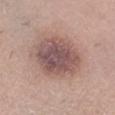Q: Was this lesion biopsied?
A: total-body-photography surveillance lesion; no biopsy
Q: What kind of image is this?
A: ~15 mm crop, total-body skin-cancer survey
Q: What lighting was used for the tile?
A: white-light
Q: Who is the patient?
A: female, in their mid-50s
Q: What did automated image analysis measure?
A: a lesion color around L≈52 a*≈19 b*≈20 in CIELAB, about 13 CIELAB-L* units darker than the surrounding skin, and a lesion-to-skin contrast of about 9 (normalized; higher = more distinct)
Q: Lesion location?
A: the right lower leg